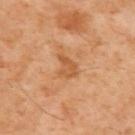Clinical impression:
This lesion was catalogued during total-body skin photography and was not selected for biopsy.
Context:
A roughly 15 mm field-of-view crop from a total-body skin photograph. The patient is a male aged 58 to 62. The lesion is on the upper back.